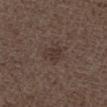{"biopsy_status": "not biopsied; imaged during a skin examination", "image": {"source": "total-body photography crop", "field_of_view_mm": 15}, "lighting": "white-light", "site": "left lower leg", "patient": {"sex": "male", "age_approx": 50}, "lesion_size": {"long_diameter_mm_approx": 3.0}}